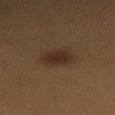This lesion was catalogued during total-body skin photography and was not selected for biopsy. Cropped from a total-body skin-imaging series; the visible field is about 15 mm. A female patient aged approximately 40. The lesion is located on the lower back.